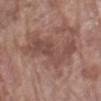No biopsy was performed on this lesion — it was imaged during a full skin examination and was not determined to be concerning.
The tile uses white-light illumination.
A roughly 15 mm field-of-view crop from a total-body skin photograph.
From the left forearm.
The lesion's longest dimension is about 8.5 mm.
A female subject approximately 70 years of age.